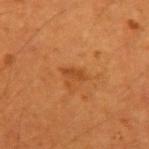Image and clinical context:
Imaged with cross-polarized lighting. A male subject, approximately 55 years of age. On the left upper arm. A roughly 15 mm field-of-view crop from a total-body skin photograph. An algorithmic analysis of the crop reported a lesion area of about 2.5 mm² and an outline eccentricity of about 0.9 (0 = round, 1 = elongated). The analysis additionally found an average lesion color of about L≈43 a*≈26 b*≈40 (CIELAB) and a normalized lesion–skin contrast near 6. The analysis additionally found an automated nevus-likeness rating near 5 out of 100. About 2.5 mm across.